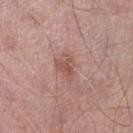| field | value |
|---|---|
| workup | imaged on a skin check; not biopsied |
| imaging modality | ~15 mm tile from a whole-body skin photo |
| patient | male, in their mid-50s |
| site | the right lower leg |
| lighting | white-light |